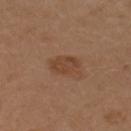lesion_size:
  long_diameter_mm_approx: 3.5
image:
  source: total-body photography crop
  field_of_view_mm: 15
lighting: white-light
site: right upper arm
patient:
  sex: female
  age_approx: 40